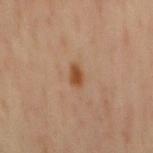The patient is a male aged 63–67. A region of skin cropped from a whole-body photographic capture, roughly 15 mm wide. The total-body-photography lesion software estimated an area of roughly 3 mm² and a shape-asymmetry score of about 0.3 (0 = symmetric). It also reported an average lesion color of about L≈47 a*≈21 b*≈34 (CIELAB), roughly 10 lightness units darker than nearby skin, and a normalized border contrast of about 9. The software also gave a border-irregularity rating of about 3/10, a color-variation rating of about 1/10, and a peripheral color-asymmetry measure near 0.5. It also reported an automated nevus-likeness rating near 95 out of 100 and a detector confidence of about 100 out of 100 that the crop contains a lesion. Approximately 2.5 mm at its widest. The lesion is on the mid back.